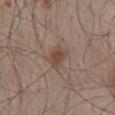biopsy status: no biopsy performed (imaged during a skin exam) | illumination: white-light illumination | TBP lesion metrics: an average lesion color of about L≈46 a*≈16 b*≈25 (CIELAB), a lesion–skin lightness drop of about 8, and a lesion-to-skin contrast of about 6.5 (normalized; higher = more distinct) | image source: ~15 mm crop, total-body skin-cancer survey | lesion diameter: about 3 mm | subject: male, aged approximately 55 | body site: the mid back.